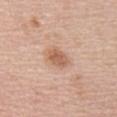Assessment:
Imaged during a routine full-body skin examination; the lesion was not biopsied and no histopathology is available.
Acquisition and patient details:
About 2.5 mm across. A female patient aged approximately 50. On the upper back. Imaged with white-light lighting. A close-up tile cropped from a whole-body skin photograph, about 15 mm across. The lesion-visualizer software estimated a detector confidence of about 100 out of 100 that the crop contains a lesion.